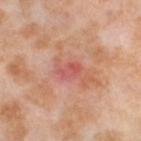Recorded during total-body skin imaging; not selected for excision or biopsy.
An algorithmic analysis of the crop reported an area of roughly 3.5 mm², a shape eccentricity near 0.75, and a shape-asymmetry score of about 0.2 (0 = symmetric). The software also gave a lesion color around L≈56 a*≈33 b*≈28 in CIELAB and a lesion–skin lightness drop of about 8. The analysis additionally found border irregularity of about 2 on a 0–10 scale and a color-variation rating of about 3.5/10. It also reported a lesion-detection confidence of about 100/100.
A female subject approximately 55 years of age.
This is a cross-polarized tile.
Located on the left thigh.
A 15 mm close-up tile from a total-body photography series done for melanoma screening.
Approximately 2.5 mm at its widest.